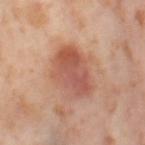On the right thigh. About 5.5 mm across. Cropped from a whole-body photographic skin survey; the tile spans about 15 mm. Imaged with cross-polarized lighting. A female subject aged approximately 55.